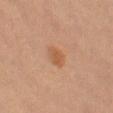Assessment:
Recorded during total-body skin imaging; not selected for excision or biopsy.